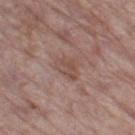Part of a total-body skin-imaging series; this lesion was reviewed on a skin check and was not flagged for biopsy. On the left thigh. Approximately 3.5 mm at its widest. The total-body-photography lesion software estimated a lesion area of about 6 mm², an eccentricity of roughly 0.65, and a shape-asymmetry score of about 0.3 (0 = symmetric). The analysis additionally found a border-irregularity rating of about 3/10, a color-variation rating of about 3/10, and peripheral color asymmetry of about 1. A female subject approximately 85 years of age or older. This is a white-light tile. A lesion tile, about 15 mm wide, cut from a 3D total-body photograph.